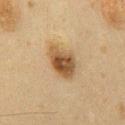A male subject, about 60 years old. Imaged with cross-polarized lighting. Located on the chest. A 15 mm close-up tile from a total-body photography series done for melanoma screening.